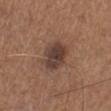The lesion was tiled from a total-body skin photograph and was not biopsied. From the left lower leg. This is a white-light tile. A region of skin cropped from a whole-body photographic capture, roughly 15 mm wide. A male subject aged around 65. About 4 mm across. An algorithmic analysis of the crop reported a detector confidence of about 100 out of 100 that the crop contains a lesion.